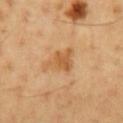biopsy status=catalogued during a skin exam; not biopsied | subject=male, approximately 50 years of age | automated metrics=an automated nevus-likeness rating near 10 out of 100 | tile lighting=cross-polarized | anatomic site=the chest | diameter=~4 mm (longest diameter) | imaging modality=~15 mm crop, total-body skin-cancer survey.